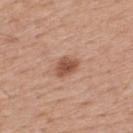Q: Was this lesion biopsied?
A: imaged on a skin check; not biopsied
Q: What is the imaging modality?
A: ~15 mm crop, total-body skin-cancer survey
Q: What is the anatomic site?
A: the upper back
Q: Patient demographics?
A: male, aged approximately 50
Q: How was the tile lit?
A: white-light
Q: What did automated image analysis measure?
A: a footprint of about 5.5 mm² and a shape-asymmetry score of about 0.25 (0 = symmetric); a lesion color around L≈53 a*≈23 b*≈30 in CIELAB, a lesion–skin lightness drop of about 12, and a normalized border contrast of about 8.5
Q: How large is the lesion?
A: about 3 mm